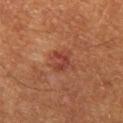This lesion was catalogued during total-body skin photography and was not selected for biopsy. A 15 mm close-up tile from a total-body photography series done for melanoma screening. From the left thigh. The lesion-visualizer software estimated a lesion–skin lightness drop of about 8 and a lesion-to-skin contrast of about 6.5 (normalized; higher = more distinct). It also reported a classifier nevus-likeness of about 15/100 and a lesion-detection confidence of about 100/100. The tile uses cross-polarized illumination. Approximately 3 mm at its widest. A male subject, roughly 60 years of age.